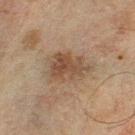Q: Was this lesion biopsied?
A: total-body-photography surveillance lesion; no biopsy
Q: Lesion size?
A: about 4.5 mm
Q: Where on the body is the lesion?
A: the left lower leg
Q: How was this image acquired?
A: ~15 mm crop, total-body skin-cancer survey
Q: Patient demographics?
A: male, aged approximately 45
Q: How was the tile lit?
A: cross-polarized illumination